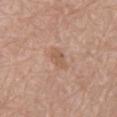Captured during whole-body skin photography for melanoma surveillance; the lesion was not biopsied.
The tile uses white-light illumination.
A 15 mm close-up tile from a total-body photography series done for melanoma screening.
Automated tile analysis of the lesion measured an area of roughly 5.5 mm². The software also gave a lesion color around L≈57 a*≈19 b*≈30 in CIELAB and roughly 7 lightness units darker than nearby skin. The analysis additionally found a border-irregularity index near 2.5/10, a within-lesion color-variation index near 2/10, and peripheral color asymmetry of about 1. The analysis additionally found an automated nevus-likeness rating near 0 out of 100 and a detector confidence of about 100 out of 100 that the crop contains a lesion.
The lesion is located on the mid back.
A male patient, approximately 70 years of age.